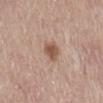The lesion was tiled from a total-body skin photograph and was not biopsied.
A female subject, aged around 65.
Cropped from a total-body skin-imaging series; the visible field is about 15 mm.
The lesion is on the abdomen.
The tile uses white-light illumination.